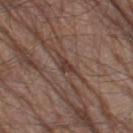The lesion was photographed on a routine skin check and not biopsied; there is no pathology result. Longest diameter approximately 2.5 mm. Captured under white-light illumination. A 15 mm close-up tile from a total-body photography series done for melanoma screening. A male subject approximately 80 years of age. On the left thigh.